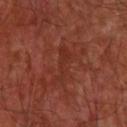This lesion was catalogued during total-body skin photography and was not selected for biopsy. The subject is a male roughly 55 years of age. Measured at roughly 4.5 mm in maximum diameter. A 15 mm close-up extracted from a 3D total-body photography capture. The total-body-photography lesion software estimated a lesion area of about 5.5 mm², an eccentricity of roughly 0.95, and two-axis asymmetry of about 0.4. The analysis additionally found an average lesion color of about L≈31 a*≈27 b*≈28 (CIELAB) and a normalized lesion–skin contrast near 5. And it measured a border-irregularity rating of about 6/10, a color-variation rating of about 0.5/10, and peripheral color asymmetry of about 0.5. The lesion is located on the upper back. This is a cross-polarized tile.